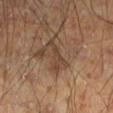Acquisition and patient details:
The tile uses cross-polarized illumination. A 15 mm close-up tile from a total-body photography series done for melanoma screening. Measured at roughly 4 mm in maximum diameter. On the left lower leg. A male subject, aged around 60.